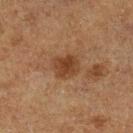Impression:
Part of a total-body skin-imaging series; this lesion was reviewed on a skin check and was not flagged for biopsy.
Context:
On the left lower leg. A male subject, roughly 75 years of age. Cropped from a whole-body photographic skin survey; the tile spans about 15 mm. Approximately 3 mm at its widest. Automated image analysis of the tile measured a lesion area of about 6.5 mm², an eccentricity of roughly 0.55, and a shape-asymmetry score of about 0.2 (0 = symmetric). And it measured a lesion color around L≈32 a*≈18 b*≈27 in CIELAB, about 8 CIELAB-L* units darker than the surrounding skin, and a lesion-to-skin contrast of about 8 (normalized; higher = more distinct). The analysis additionally found border irregularity of about 2 on a 0–10 scale, a color-variation rating of about 2.5/10, and peripheral color asymmetry of about 1.